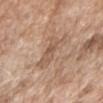Imaged during a routine full-body skin examination; the lesion was not biopsied and no histopathology is available.
A 15 mm close-up extracted from a 3D total-body photography capture.
The subject is a female in their mid-70s.
Imaged with white-light lighting.
On the arm.